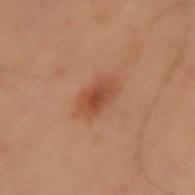Clinical impression: Recorded during total-body skin imaging; not selected for excision or biopsy.